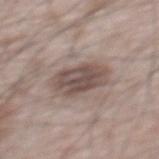subject = male, approximately 65 years of age; diameter = ≈5 mm; anatomic site = the mid back; image = ~15 mm tile from a whole-body skin photo; tile lighting = white-light illumination.